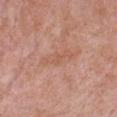Case summary:
* biopsy status: catalogued during a skin exam; not biopsied
* automated lesion analysis: border irregularity of about 4.5 on a 0–10 scale and a peripheral color-asymmetry measure near 0.5; a classifier nevus-likeness of about 0/100 and a lesion-detection confidence of about 100/100
* tile lighting: white-light illumination
* site: the chest
* lesion diameter: ~4.5 mm (longest diameter)
* image: ~15 mm crop, total-body skin-cancer survey
* patient: female, in their 60s